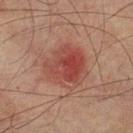biopsy status: no biopsy performed (imaged during a skin exam)
size: about 5 mm
imaging modality: total-body-photography crop, ~15 mm field of view
subject: male, approximately 75 years of age
site: the left lower leg
automated lesion analysis: a within-lesion color-variation index near 7/10 and radial color variation of about 2.5